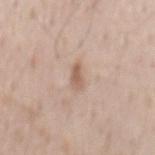Clinical impression: The lesion was photographed on a routine skin check and not biopsied; there is no pathology result. Context: From the mid back. Approximately 3 mm at its widest. The lesion-visualizer software estimated a mean CIELAB color near L≈59 a*≈18 b*≈28, about 10 CIELAB-L* units darker than the surrounding skin, and a lesion-to-skin contrast of about 7 (normalized; higher = more distinct). And it measured a border-irregularity index near 3/10, internal color variation of about 0.5 on a 0–10 scale, and a peripheral color-asymmetry measure near 0. A male patient in their 50s. A lesion tile, about 15 mm wide, cut from a 3D total-body photograph.